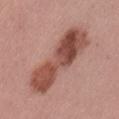illumination: white-light | automated lesion analysis: an area of roughly 27 mm² and an eccentricity of roughly 0.95; a mean CIELAB color near L≈49 a*≈24 b*≈26, a lesion–skin lightness drop of about 14, and a lesion-to-skin contrast of about 9.5 (normalized; higher = more distinct); an automated nevus-likeness rating near 5 out of 100 | patient: female, in their mid- to late 50s | imaging modality: ~15 mm crop, total-body skin-cancer survey | site: the lower back.